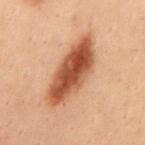The lesion was tiled from a total-body skin photograph and was not biopsied.
Approximately 8.5 mm at its widest.
The tile uses cross-polarized illumination.
The lesion is located on the mid back.
A close-up tile cropped from a whole-body skin photograph, about 15 mm across.
The total-body-photography lesion software estimated border irregularity of about 2.5 on a 0–10 scale, a within-lesion color-variation index near 6.5/10, and radial color variation of about 2. The analysis additionally found a nevus-likeness score of about 95/100 and a lesion-detection confidence of about 100/100.
The patient is a male aged 28 to 32.